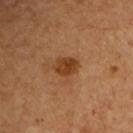This lesion was catalogued during total-body skin photography and was not selected for biopsy. The recorded lesion diameter is about 3 mm. The patient is a male about 50 years old. The tile uses cross-polarized illumination. This image is a 15 mm lesion crop taken from a total-body photograph. Located on the chest.